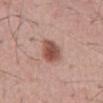follow-up = imaged on a skin check; not biopsied | subject = male, roughly 55 years of age | anatomic site = the back | acquisition = 15 mm crop, total-body photography.